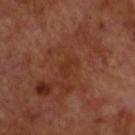{
  "biopsy_status": "not biopsied; imaged during a skin examination",
  "lighting": "cross-polarized",
  "site": "head or neck",
  "lesion_size": {
    "long_diameter_mm_approx": 3.0
  },
  "patient": {
    "sex": "male",
    "age_approx": 60
  },
  "image": {
    "source": "total-body photography crop",
    "field_of_view_mm": 15
  }
}